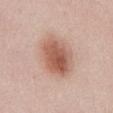| feature | finding |
|---|---|
| workup | no biopsy performed (imaged during a skin exam) |
| tile lighting | white-light |
| automated metrics | an area of roughly 18 mm², an eccentricity of roughly 0.6, and a symmetry-axis asymmetry near 0.15; a classifier nevus-likeness of about 100/100 and a lesion-detection confidence of about 100/100 |
| size | about 5.5 mm |
| body site | the abdomen |
| acquisition | ~15 mm tile from a whole-body skin photo |
| patient | female, in their 40s |